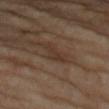biopsy_status: not biopsied; imaged during a skin examination
lesion_size:
  long_diameter_mm_approx: 3.0
image:
  source: total-body photography crop
  field_of_view_mm: 15
lighting: cross-polarized
site: left forearm
automated_metrics:
  area_mm2_approx: 3.5
  eccentricity: 0.85
  shape_asymmetry: 0.5
  color_variation_0_10: 1.0
  peripheral_color_asymmetry: 0.5
patient:
  sex: female
  age_approx: 70